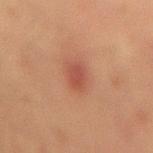This lesion was catalogued during total-body skin photography and was not selected for biopsy.
This image is a 15 mm lesion crop taken from a total-body photograph.
A male patient aged around 45.
Captured under cross-polarized illumination.
Longest diameter approximately 3 mm.
The lesion is located on the lower back.
Automated tile analysis of the lesion measured a mean CIELAB color near L≈41 a*≈24 b*≈26, about 8 CIELAB-L* units darker than the surrounding skin, and a normalized border contrast of about 6.5. And it measured a nevus-likeness score of about 75/100.